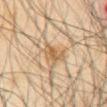follow-up: no biopsy performed (imaged during a skin exam)
automated metrics: a border-irregularity index near 4.5/10, a color-variation rating of about 3.5/10, and peripheral color asymmetry of about 1
site: the chest
tile lighting: cross-polarized illumination
patient: male, approximately 65 years of age
acquisition: ~15 mm tile from a whole-body skin photo
diameter: about 4 mm